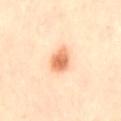{
  "biopsy_status": "not biopsied; imaged during a skin examination",
  "patient": {
    "sex": "female",
    "age_approx": 40
  },
  "site": "lower back",
  "image": {
    "source": "total-body photography crop",
    "field_of_view_mm": 15
  },
  "lighting": "cross-polarized",
  "lesion_size": {
    "long_diameter_mm_approx": 3.0
  }
}